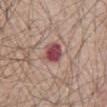Clinical impression:
Imaged during a routine full-body skin examination; the lesion was not biopsied and no histopathology is available.
Context:
A roughly 15 mm field-of-view crop from a total-body skin photograph. A male subject aged around 65. Automated image analysis of the tile measured a shape eccentricity near 0.55. The software also gave border irregularity of about 2 on a 0–10 scale, internal color variation of about 5 on a 0–10 scale, and radial color variation of about 1.5. The analysis additionally found an automated nevus-likeness rating near 5 out of 100 and lesion-presence confidence of about 100/100. Located on the abdomen. The tile uses white-light illumination.